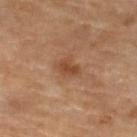Part of a total-body skin-imaging series; this lesion was reviewed on a skin check and was not flagged for biopsy. Located on the left lower leg. Captured under cross-polarized illumination. Cropped from a whole-body photographic skin survey; the tile spans about 15 mm. A female subject about 70 years old. Approximately 2.5 mm at its widest.